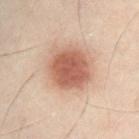{
  "biopsy_status": "not biopsied; imaged during a skin examination",
  "patient": {
    "sex": "male",
    "age_approx": 50
  },
  "lighting": "cross-polarized",
  "image": {
    "source": "total-body photography crop",
    "field_of_view_mm": 15
  },
  "automated_metrics": {
    "area_mm2_approx": 17.0,
    "eccentricity": 0.35,
    "shape_asymmetry": 0.1,
    "cielab_L": 46,
    "cielab_a": 19,
    "cielab_b": 25,
    "vs_skin_contrast_norm": 9.0,
    "border_irregularity_0_10": 1.0,
    "color_variation_0_10": 3.0
  },
  "lesion_size": {
    "long_diameter_mm_approx": 4.5
  },
  "site": "front of the torso"
}